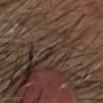Q: Is there a histopathology result?
A: catalogued during a skin exam; not biopsied
Q: Automated lesion metrics?
A: a shape eccentricity near 0.85 and a symmetry-axis asymmetry near 0.35; border irregularity of about 2.5 on a 0–10 scale and peripheral color asymmetry of about 0
Q: Where on the body is the lesion?
A: the head or neck
Q: What kind of image is this?
A: ~15 mm tile from a whole-body skin photo
Q: How large is the lesion?
A: ~1 mm (longest diameter)
Q: Who is the patient?
A: male, aged approximately 65
Q: What lighting was used for the tile?
A: cross-polarized illumination